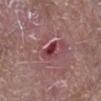| feature | finding |
|---|---|
| biopsy status | total-body-photography surveillance lesion; no biopsy |
| automated lesion analysis | an automated nevus-likeness rating near 0 out of 100 and a lesion-detection confidence of about 95/100 |
| tile lighting | white-light illumination |
| image | ~15 mm tile from a whole-body skin photo |
| patient | male, aged approximately 65 |
| anatomic site | the right lower leg |